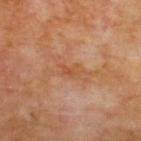Assessment: Captured during whole-body skin photography for melanoma surveillance; the lesion was not biopsied. Clinical summary: From the upper back. Captured under cross-polarized illumination. A close-up tile cropped from a whole-body skin photograph, about 15 mm across. A male patient aged around 70. Approximately 3 mm at its widest.